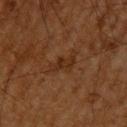Captured during whole-body skin photography for melanoma surveillance; the lesion was not biopsied. Located on the head or neck. About 4.5 mm across. The patient is a male roughly 65 years of age. A region of skin cropped from a whole-body photographic capture, roughly 15 mm wide. The tile uses cross-polarized illumination.